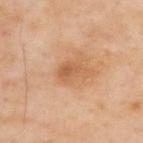<tbp_lesion>
<biopsy_status>not biopsied; imaged during a skin examination</biopsy_status>
<lighting>cross-polarized</lighting>
<patient>
  <sex>male</sex>
  <age_approx>55</age_approx>
</patient>
<site>upper back</site>
<image>
  <source>total-body photography crop</source>
  <field_of_view_mm>15</field_of_view_mm>
</image>
<lesion_size>
  <long_diameter_mm_approx>3.5</long_diameter_mm_approx>
</lesion_size>
</tbp_lesion>